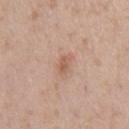A lesion tile, about 15 mm wide, cut from a 3D total-body photograph. A male patient about 55 years old. On the front of the torso. Captured under white-light illumination. Approximately 2.5 mm at its widest. An algorithmic analysis of the crop reported a shape eccentricity near 0.85 and a symmetry-axis asymmetry near 0.2. The analysis additionally found an average lesion color of about L≈58 a*≈20 b*≈30 (CIELAB) and roughly 8 lightness units darker than nearby skin. And it measured a color-variation rating of about 2.5/10 and peripheral color asymmetry of about 1. And it measured a classifier nevus-likeness of about 10/100 and a detector confidence of about 100 out of 100 that the crop contains a lesion.